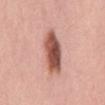Clinical impression: Part of a total-body skin-imaging series; this lesion was reviewed on a skin check and was not flagged for biopsy. Image and clinical context: A region of skin cropped from a whole-body photographic capture, roughly 15 mm wide. From the back. Longest diameter approximately 6 mm. The patient is a female roughly 55 years of age.